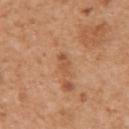{"lesion_size": {"long_diameter_mm_approx": 3.0}, "patient": {"sex": "male", "age_approx": 55}, "lighting": "white-light", "site": "right upper arm", "image": {"source": "total-body photography crop", "field_of_view_mm": 15}}